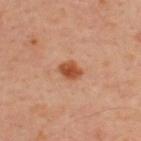Q: Is there a histopathology result?
A: catalogued during a skin exam; not biopsied
Q: What did automated image analysis measure?
A: an average lesion color of about L≈50 a*≈27 b*≈36 (CIELAB), a lesion–skin lightness drop of about 12, and a normalized border contrast of about 9.5; border irregularity of about 1.5 on a 0–10 scale, internal color variation of about 3 on a 0–10 scale, and a peripheral color-asymmetry measure near 1; a nevus-likeness score of about 95/100 and a lesion-detection confidence of about 100/100
Q: Illumination type?
A: cross-polarized illumination
Q: Lesion size?
A: about 2.5 mm
Q: Patient demographics?
A: male, approximately 45 years of age
Q: What kind of image is this?
A: total-body-photography crop, ~15 mm field of view
Q: What is the anatomic site?
A: the upper back